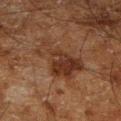biopsy status=no biopsy performed (imaged during a skin exam) | subject=male, in their 60s | lesion size=about 6 mm | anatomic site=the leg | imaging modality=total-body-photography crop, ~15 mm field of view | image-analysis metrics=a shape eccentricity near 0.8; a lesion color around L≈26 a*≈17 b*≈23 in CIELAB, about 7 CIELAB-L* units darker than the surrounding skin, and a normalized border contrast of about 7.5; a detector confidence of about 100 out of 100 that the crop contains a lesion | lighting=cross-polarized illumination.